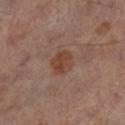Clinical impression: The lesion was tiled from a total-body skin photograph and was not biopsied. Image and clinical context: The lesion is on the left lower leg. A region of skin cropped from a whole-body photographic capture, roughly 15 mm wide. A male subject aged around 65. Imaged with cross-polarized lighting.